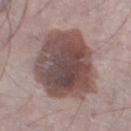The lesion was tiled from a total-body skin photograph and was not biopsied.
The subject is a male roughly 70 years of age.
The lesion is on the left lower leg.
A 15 mm close-up tile from a total-body photography series done for melanoma screening.